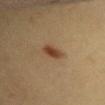Image and clinical context: An algorithmic analysis of the crop reported an area of roughly 5 mm², a shape eccentricity near 0.8, and a symmetry-axis asymmetry near 0.25. The software also gave a within-lesion color-variation index near 3/10 and peripheral color asymmetry of about 1. And it measured a classifier nevus-likeness of about 100/100 and a detector confidence of about 100 out of 100 that the crop contains a lesion. The tile uses cross-polarized illumination. A roughly 15 mm field-of-view crop from a total-body skin photograph. Measured at roughly 3 mm in maximum diameter. The patient is a female in their 30s. The lesion is located on the chest.